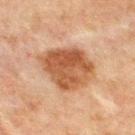biopsy_status: not biopsied; imaged during a skin examination
site: chest
automated_metrics:
  area_mm2_approx: 24.0
  eccentricity: 0.55
  shape_asymmetry: 0.2
  cielab_L: 44
  cielab_a: 20
  cielab_b: 31
  vs_skin_darker_L: 11.0
  vs_skin_contrast_norm: 9.5
  border_irregularity_0_10: 2.0
  color_variation_0_10: 5.0
  peripheral_color_asymmetry: 2.0
  nevus_likeness_0_100: 40
lesion_size:
  long_diameter_mm_approx: 6.0
image:
  source: total-body photography crop
  field_of_view_mm: 15
patient:
  sex: male
  age_approx: 65
lighting: cross-polarized